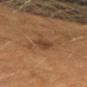<tbp_lesion>
<biopsy_status>not biopsied; imaged during a skin examination</biopsy_status>
<site>left forearm</site>
<patient>
  <sex>male</sex>
  <age_approx>40</age_approx>
</patient>
<image>
  <source>total-body photography crop</source>
  <field_of_view_mm>15</field_of_view_mm>
</image>
<lesion_size>
  <long_diameter_mm_approx>3.5</long_diameter_mm_approx>
</lesion_size>
<automated_metrics>
  <border_irregularity_0_10>3.0</border_irregularity_0_10>
  <color_variation_0_10>1.5</color_variation_0_10>
  <peripheral_color_asymmetry>0.5</peripheral_color_asymmetry>
  <lesion_detection_confidence_0_100>100</lesion_detection_confidence_0_100>
</automated_metrics>
<lighting>cross-polarized</lighting>
</tbp_lesion>